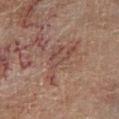Q: Was a biopsy performed?
A: no biopsy performed (imaged during a skin exam)